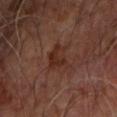biopsy status=no biopsy performed (imaged during a skin exam)
size=≈3.5 mm
subject=male, aged 63 to 67
illumination=cross-polarized
site=the left forearm
image source=total-body-photography crop, ~15 mm field of view